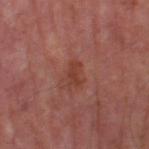biopsy status: catalogued during a skin exam; not biopsied | TBP lesion metrics: a footprint of about 3.5 mm², an eccentricity of roughly 0.85, and a shape-asymmetry score of about 0.3 (0 = symmetric) | diameter: ≈2.5 mm | image source: ~15 mm tile from a whole-body skin photo | anatomic site: the left thigh | subject: male, aged 63 to 67 | illumination: cross-polarized.